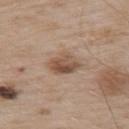  biopsy_status: not biopsied; imaged during a skin examination
  image:
    source: total-body photography crop
    field_of_view_mm: 15
  site: upper back
  patient:
    sex: male
    age_approx: 55